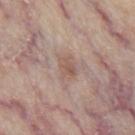* workup — imaged on a skin check; not biopsied
* tile lighting — white-light illumination
* subject — male, roughly 65 years of age
* imaging modality — 15 mm crop, total-body photography
* lesion diameter — ≈2.5 mm
* site — the abdomen